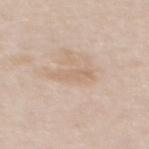No biopsy was performed on this lesion — it was imaged during a full skin examination and was not determined to be concerning. A 15 mm crop from a total-body photograph taken for skin-cancer surveillance. Located on the mid back. The subject is a female aged 43 to 47. Measured at roughly 4.5 mm in maximum diameter. Captured under white-light illumination.